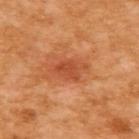workup: catalogued during a skin exam; not biopsied
acquisition: ~15 mm crop, total-body skin-cancer survey
location: the upper back
image-analysis metrics: an average lesion color of about L≈52 a*≈33 b*≈41 (CIELAB), about 9 CIELAB-L* units darker than the surrounding skin, and a normalized lesion–skin contrast near 6.5; a border-irregularity rating of about 3.5/10, internal color variation of about 2.5 on a 0–10 scale, and peripheral color asymmetry of about 1; an automated nevus-likeness rating near 0 out of 100 and a detector confidence of about 100 out of 100 that the crop contains a lesion
lesion size: about 3.5 mm
tile lighting: cross-polarized
subject: female, about 55 years old